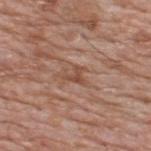notes = no biopsy performed (imaged during a skin exam)
size = ~2.5 mm (longest diameter)
site = the upper back
image source = ~15 mm tile from a whole-body skin photo
subject = male, aged 58 to 62
lighting = white-light illumination
automated metrics = a lesion area of about 3 mm²; a classifier nevus-likeness of about 0/100 and a lesion-detection confidence of about 95/100